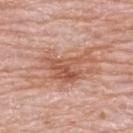- workup · imaged on a skin check; not biopsied
- subject · female, in their mid- to late 60s
- imaging modality · 15 mm crop, total-body photography
- image-analysis metrics · an outline eccentricity of about 0.75 (0 = round, 1 = elongated) and a symmetry-axis asymmetry near 0.5; about 10 CIELAB-L* units darker than the surrounding skin and a lesion-to-skin contrast of about 7.5 (normalized; higher = more distinct); a border-irregularity rating of about 7.5/10, a within-lesion color-variation index near 5.5/10, and a peripheral color-asymmetry measure near 2; an automated nevus-likeness rating near 0 out of 100
- site · the upper back